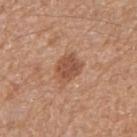<lesion>
<biopsy_status>not biopsied; imaged during a skin examination</biopsy_status>
<patient>
  <sex>female</sex>
  <age_approx>50</age_approx>
</patient>
<lighting>white-light</lighting>
<image>
  <source>total-body photography crop</source>
  <field_of_view_mm>15</field_of_view_mm>
</image>
<lesion_size>
  <long_diameter_mm_approx>3.5</long_diameter_mm_approx>
</lesion_size>
<site>right forearm</site>
</lesion>